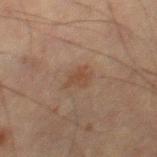Impression: Recorded during total-body skin imaging; not selected for excision or biopsy. Image and clinical context: Cropped from a total-body skin-imaging series; the visible field is about 15 mm. The recorded lesion diameter is about 2.5 mm. The lesion-visualizer software estimated a lesion area of about 3.5 mm², an outline eccentricity of about 0.75 (0 = round, 1 = elongated), and two-axis asymmetry of about 0.4. The patient is a male aged around 65. The lesion is located on the left thigh. This is a cross-polarized tile.